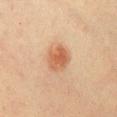follow-up=imaged on a skin check; not biopsied
patient=female, aged around 45
acquisition=~15 mm tile from a whole-body skin photo
site=the chest